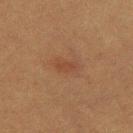Assessment:
The lesion was photographed on a routine skin check and not biopsied; there is no pathology result.
Image and clinical context:
Automated tile analysis of the lesion measured a shape-asymmetry score of about 0.25 (0 = symmetric). The software also gave a border-irregularity rating of about 2/10, internal color variation of about 1 on a 0–10 scale, and a peripheral color-asymmetry measure near 0.5. On the leg. Cropped from a whole-body photographic skin survey; the tile spans about 15 mm. Captured under cross-polarized illumination. A female subject about 40 years old.